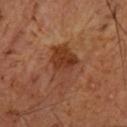biopsy_status: not biopsied; imaged during a skin examination
automated_metrics:
  area_mm2_approx: 12.0
  eccentricity: 0.8
  shape_asymmetry: 0.35
  border_irregularity_0_10: 4.5
  color_variation_0_10: 4.5
patient:
  sex: male
  age_approx: 50
image:
  source: total-body photography crop
  field_of_view_mm: 15
site: arm